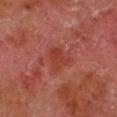Assessment:
This lesion was catalogued during total-body skin photography and was not selected for biopsy.
Acquisition and patient details:
The lesion is on the right lower leg. A 15 mm crop from a total-body photograph taken for skin-cancer surveillance. Captured under cross-polarized illumination. The patient is a male aged around 80.